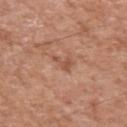Captured during whole-body skin photography for melanoma surveillance; the lesion was not biopsied. A region of skin cropped from a whole-body photographic capture, roughly 15 mm wide. Located on the left upper arm. A male patient, aged approximately 55. The total-body-photography lesion software estimated a lesion area of about 2.5 mm², an outline eccentricity of about 0.8 (0 = round, 1 = elongated), and a shape-asymmetry score of about 0.65 (0 = symmetric). The analysis additionally found a lesion-to-skin contrast of about 6 (normalized; higher = more distinct). The analysis additionally found a classifier nevus-likeness of about 0/100 and lesion-presence confidence of about 100/100. About 2.5 mm across. Imaged with white-light lighting.